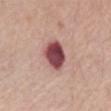Cropped from a whole-body photographic skin survey; the tile spans about 15 mm.
Automated tile analysis of the lesion measured a shape eccentricity near 0.6 and a shape-asymmetry score of about 0.15 (0 = symmetric). It also reported a lesion-detection confidence of about 100/100.
The subject is a female aged around 75.
Captured under white-light illumination.
Located on the abdomen.
Approximately 4 mm at its widest.